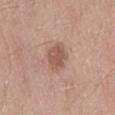Recorded during total-body skin imaging; not selected for excision or biopsy. A roughly 15 mm field-of-view crop from a total-body skin photograph. The lesion is located on the lower back. A male patient aged 38 to 42.